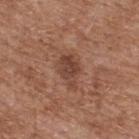The lesion was tiled from a total-body skin photograph and was not biopsied. A male subject aged 73–77. This image is a 15 mm lesion crop taken from a total-body photograph. The lesion is located on the upper back. Approximately 4 mm at its widest.